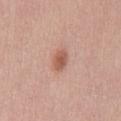Q: Is there a histopathology result?
A: no biopsy performed (imaged during a skin exam)
Q: What kind of image is this?
A: ~15 mm tile from a whole-body skin photo
Q: Patient demographics?
A: female, about 55 years old
Q: Where on the body is the lesion?
A: the mid back
Q: Illumination type?
A: white-light
Q: What is the lesion's diameter?
A: about 3 mm